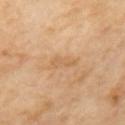This lesion was catalogued during total-body skin photography and was not selected for biopsy. From the back. Captured under cross-polarized illumination. Cropped from a whole-body photographic skin survey; the tile spans about 15 mm.